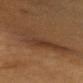workup — catalogued during a skin exam; not biopsied
acquisition — ~15 mm crop, total-body skin-cancer survey
anatomic site — the chest
illumination — cross-polarized illumination
subject — female, aged 53 to 57